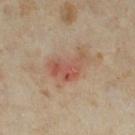notes: no biopsy performed (imaged during a skin exam); subject: female, in their mid- to late 30s; image: ~15 mm crop, total-body skin-cancer survey; illumination: cross-polarized; location: the right thigh.